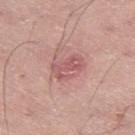Clinical impression:
No biopsy was performed on this lesion — it was imaged during a full skin examination and was not determined to be concerning.
Acquisition and patient details:
A male patient, aged around 70. A region of skin cropped from a whole-body photographic capture, roughly 15 mm wide. The lesion-visualizer software estimated a footprint of about 7 mm², an eccentricity of roughly 0.8, and two-axis asymmetry of about 0.3. And it measured a lesion color around L≈56 a*≈27 b*≈22 in CIELAB, roughly 9 lightness units darker than nearby skin, and a normalized lesion–skin contrast near 6. And it measured border irregularity of about 4 on a 0–10 scale, internal color variation of about 2.5 on a 0–10 scale, and peripheral color asymmetry of about 1. And it measured an automated nevus-likeness rating near 0 out of 100 and lesion-presence confidence of about 100/100. This is a white-light tile. The lesion is on the left thigh. The lesion's longest dimension is about 4 mm.